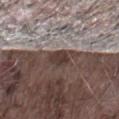Q: Was this lesion biopsied?
A: catalogued during a skin exam; not biopsied
Q: What kind of image is this?
A: ~15 mm tile from a whole-body skin photo
Q: Patient demographics?
A: male, aged around 75
Q: Where on the body is the lesion?
A: the right thigh
Q: How was the tile lit?
A: white-light illumination
Q: How large is the lesion?
A: ~2.5 mm (longest diameter)